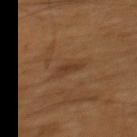Imaged during a routine full-body skin examination; the lesion was not biopsied and no histopathology is available. Imaged with cross-polarized lighting. A lesion tile, about 15 mm wide, cut from a 3D total-body photograph. The recorded lesion diameter is about 2.5 mm. A male patient aged around 60. From the upper back.